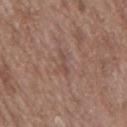<tbp_lesion>
<biopsy_status>not biopsied; imaged during a skin examination</biopsy_status>
<automated_metrics>
  <area_mm2_approx>2.5</area_mm2_approx>
  <eccentricity>0.9</eccentricity>
  <border_irregularity_0_10>5.5</border_irregularity_0_10>
  <color_variation_0_10>0.0</color_variation_0_10>
  <peripheral_color_asymmetry>0.0</peripheral_color_asymmetry>
  <nevus_likeness_0_100>0</nevus_likeness_0_100>
  <lesion_detection_confidence_0_100>80</lesion_detection_confidence_0_100>
</automated_metrics>
<lighting>white-light</lighting>
<patient>
  <sex>male</sex>
  <age_approx>70</age_approx>
</patient>
<site>mid back</site>
<image>
  <source>total-body photography crop</source>
  <field_of_view_mm>15</field_of_view_mm>
</image>
</tbp_lesion>